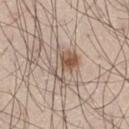A lesion tile, about 15 mm wide, cut from a 3D total-body photograph. From the left thigh. A male subject, approximately 30 years of age.